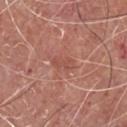The lesion was photographed on a routine skin check and not biopsied; there is no pathology result. Longest diameter approximately 2.5 mm. A 15 mm close-up extracted from a 3D total-body photography capture. A male patient in their mid-60s. Imaged with white-light lighting. From the chest.